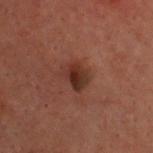Case summary:
– follow-up: catalogued during a skin exam; not biopsied